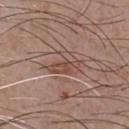biopsy status: total-body-photography surveillance lesion; no biopsy | automated lesion analysis: a lesion area of about 6 mm² and an outline eccentricity of about 0.8 (0 = round, 1 = elongated) | image: 15 mm crop, total-body photography | illumination: white-light | subject: male, in their mid-50s | location: the chest | diameter: about 3.5 mm.